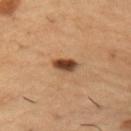Part of a total-body skin-imaging series; this lesion was reviewed on a skin check and was not flagged for biopsy. A region of skin cropped from a whole-body photographic capture, roughly 15 mm wide. The lesion is located on the right upper arm. The tile uses cross-polarized illumination. Approximately 3 mm at its widest. A male subject aged 53–57.